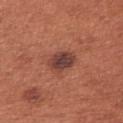Impression: Part of a total-body skin-imaging series; this lesion was reviewed on a skin check and was not flagged for biopsy. Background: Located on the right upper arm. The lesion-visualizer software estimated an area of roughly 7.5 mm² and a symmetry-axis asymmetry near 0.15. And it measured a border-irregularity index near 2/10 and internal color variation of about 5 on a 0–10 scale. It also reported an automated nevus-likeness rating near 100 out of 100 and lesion-presence confidence of about 100/100. About 3.5 mm across. A lesion tile, about 15 mm wide, cut from a 3D total-body photograph. A female subject, aged around 65.